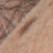Captured during whole-body skin photography for melanoma surveillance; the lesion was not biopsied.